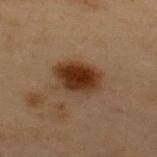Context:
A roughly 15 mm field-of-view crop from a total-body skin photograph. The lesion is located on the upper back. A male patient aged 53–57.